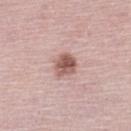Q: Was a biopsy performed?
A: imaged on a skin check; not biopsied
Q: What is the lesion's diameter?
A: about 3 mm
Q: What is the imaging modality?
A: total-body-photography crop, ~15 mm field of view
Q: What did automated image analysis measure?
A: a classifier nevus-likeness of about 80/100 and a detector confidence of about 100 out of 100 that the crop contains a lesion
Q: What lighting was used for the tile?
A: white-light illumination
Q: Who is the patient?
A: female, aged 63–67
Q: Lesion location?
A: the left lower leg